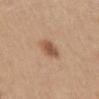Impression:
Recorded during total-body skin imaging; not selected for excision or biopsy.